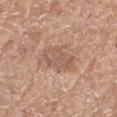Context:
The recorded lesion diameter is about 5 mm. From the left thigh. The patient is a female aged around 75. Imaged with white-light lighting. A lesion tile, about 15 mm wide, cut from a 3D total-body photograph.